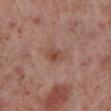  biopsy_status: not biopsied; imaged during a skin examination
  image:
    source: total-body photography crop
    field_of_view_mm: 15
  site: right lower leg
  automated_metrics:
    border_irregularity_0_10: 2.0
    lesion_detection_confidence_0_100: 100
  lesion_size:
    long_diameter_mm_approx: 3.0
  patient:
    sex: male
    age_approx: 70